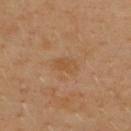Q: Was a biopsy performed?
A: no biopsy performed (imaged during a skin exam)
Q: How large is the lesion?
A: ~3 mm (longest diameter)
Q: What are the patient's age and sex?
A: male, aged around 40
Q: What is the imaging modality?
A: ~15 mm crop, total-body skin-cancer survey
Q: Where on the body is the lesion?
A: the upper back
Q: What did automated image analysis measure?
A: an area of roughly 4.5 mm² and a symmetry-axis asymmetry near 0.25; a lesion color around L≈51 a*≈20 b*≈36 in CIELAB, a lesion–skin lightness drop of about 5, and a normalized border contrast of about 5; a border-irregularity index near 2/10, a within-lesion color-variation index near 1.5/10, and radial color variation of about 0.5; an automated nevus-likeness rating near 0 out of 100 and a detector confidence of about 100 out of 100 that the crop contains a lesion
Q: What lighting was used for the tile?
A: cross-polarized